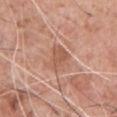Impression: The lesion was tiled from a total-body skin photograph and was not biopsied. Background: The patient is a male aged approximately 60. From the front of the torso. A 15 mm crop from a total-body photograph taken for skin-cancer surveillance.